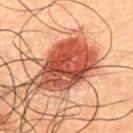biopsy status: total-body-photography surveillance lesion; no biopsy | subject: male, roughly 50 years of age | location: the front of the torso | tile lighting: cross-polarized | automated metrics: a lesion color around L≈38 a*≈26 b*≈28 in CIELAB, about 15 CIELAB-L* units darker than the surrounding skin, and a normalized border contrast of about 12; a lesion-detection confidence of about 100/100 | image: total-body-photography crop, ~15 mm field of view | size: ≈7.5 mm.